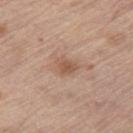<case>
<biopsy_status>not biopsied; imaged during a skin examination</biopsy_status>
<lighting>white-light</lighting>
<lesion_size>
  <long_diameter_mm_approx>2.5</long_diameter_mm_approx>
</lesion_size>
<patient>
  <sex>male</sex>
  <age_approx>70</age_approx>
</patient>
<image>
  <source>total-body photography crop</source>
  <field_of_view_mm>15</field_of_view_mm>
</image>
<site>right thigh</site>
<automated_metrics>
  <area_mm2_approx>3.5</area_mm2_approx>
  <eccentricity>0.7</eccentricity>
  <shape_asymmetry>0.35</shape_asymmetry>
  <cielab_L>54</cielab_L>
  <cielab_a>20</cielab_a>
  <cielab_b>29</cielab_b>
  <vs_skin_darker_L>9.0</vs_skin_darker_L>
  <vs_skin_contrast_norm>6.5</vs_skin_contrast_norm>
  <border_irregularity_0_10>3.0</border_irregularity_0_10>
  <color_variation_0_10>2.0</color_variation_0_10>
  <lesion_detection_confidence_0_100>100</lesion_detection_confidence_0_100>
</automated_metrics>
</case>